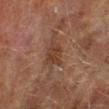Clinical impression: The lesion was photographed on a routine skin check and not biopsied; there is no pathology result. Acquisition and patient details: Located on the left lower leg. Captured under cross-polarized illumination. The subject is a male aged around 75. A 15 mm close-up extracted from a 3D total-body photography capture.